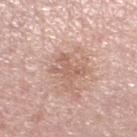<record>
  <lighting>white-light</lighting>
  <site>left lower leg</site>
  <lesion_size>
    <long_diameter_mm_approx>3.0</long_diameter_mm_approx>
  </lesion_size>
  <automated_metrics>
    <cielab_L>60</cielab_L>
    <cielab_a>21</cielab_a>
    <cielab_b>27</cielab_b>
    <vs_skin_darker_L>8.0</vs_skin_darker_L>
    <vs_skin_contrast_norm>5.5</vs_skin_contrast_norm>
    <border_irregularity_0_10>7.0</border_irregularity_0_10>
    <peripheral_color_asymmetry>0.5</peripheral_color_asymmetry>
    <nevus_likeness_0_100>0</nevus_likeness_0_100>
    <lesion_detection_confidence_0_100>100</lesion_detection_confidence_0_100>
  </automated_metrics>
  <image>
    <source>total-body photography crop</source>
    <field_of_view_mm>15</field_of_view_mm>
  </image>
  <patient>
    <sex>male</sex>
    <age_approx>60</age_approx>
  </patient>
</record>